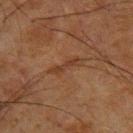Q: Is there a histopathology result?
A: no biopsy performed (imaged during a skin exam)
Q: What are the patient's age and sex?
A: male, aged 63–67
Q: Where on the body is the lesion?
A: the back
Q: How was the tile lit?
A: cross-polarized illumination
Q: What is the imaging modality?
A: ~15 mm tile from a whole-body skin photo
Q: Automated lesion metrics?
A: a lesion area of about 5 mm², an outline eccentricity of about 0.95 (0 = round, 1 = elongated), and a symmetry-axis asymmetry near 0.4